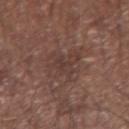lesion diameter: ≈5 mm | anatomic site: the left upper arm | subject: male, roughly 65 years of age | tile lighting: white-light | image: ~15 mm tile from a whole-body skin photo | TBP lesion metrics: a mean CIELAB color near L≈38 a*≈18 b*≈22, about 6 CIELAB-L* units darker than the surrounding skin, and a normalized lesion–skin contrast near 5.